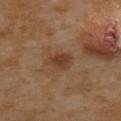Assessment: No biopsy was performed on this lesion — it was imaged during a full skin examination and was not determined to be concerning. Background: A 15 mm crop from a total-body photograph taken for skin-cancer surveillance. A female patient, roughly 55 years of age. Located on the back.